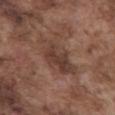The lesion was photographed on a routine skin check and not biopsied; there is no pathology result.
The lesion is located on the front of the torso.
The subject is a male aged around 75.
A lesion tile, about 15 mm wide, cut from a 3D total-body photograph.